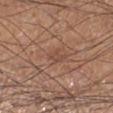patient = male, roughly 55 years of age; acquisition = ~15 mm tile from a whole-body skin photo; anatomic site = the right lower leg.